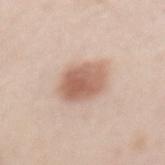<case>
<biopsy_status>not biopsied; imaged during a skin examination</biopsy_status>
</case>